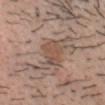  lesion_size:
    long_diameter_mm_approx: 4.0
  site: head or neck
  patient:
    sex: male
    age_approx: 25
  automated_metrics:
    lesion_detection_confidence_0_100: 95
  image:
    source: total-body photography crop
    field_of_view_mm: 15
  lighting: white-light